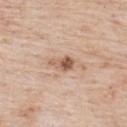Imaged during a routine full-body skin examination; the lesion was not biopsied and no histopathology is available.
The lesion is on the upper back.
A close-up tile cropped from a whole-body skin photograph, about 15 mm across.
A female patient aged around 70.
Measured at roughly 3 mm in maximum diameter.
Automated image analysis of the tile measured an average lesion color of about L≈58 a*≈19 b*≈30 (CIELAB), roughly 13 lightness units darker than nearby skin, and a lesion-to-skin contrast of about 8.5 (normalized; higher = more distinct). The analysis additionally found a nevus-likeness score of about 70/100 and lesion-presence confidence of about 100/100.